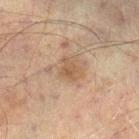| key | value |
|---|---|
| biopsy status | catalogued during a skin exam; not biopsied |
| size | about 2.5 mm |
| automated lesion analysis | an average lesion color of about L≈47 a*≈15 b*≈28 (CIELAB) and roughly 7 lightness units darker than nearby skin; a border-irregularity index near 3/10 |
| image source | ~15 mm crop, total-body skin-cancer survey |
| site | the right lower leg |
| subject | male, in their mid-70s |
| tile lighting | cross-polarized illumination |